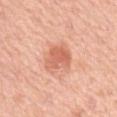This lesion was catalogued during total-body skin photography and was not selected for biopsy.
A roughly 15 mm field-of-view crop from a total-body skin photograph.
Longest diameter approximately 3.5 mm.
From the arm.
A female patient aged 48–52.